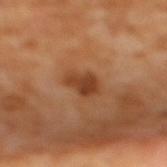image: ~15 mm tile from a whole-body skin photo; location: the upper back; illumination: cross-polarized; subject: female, about 55 years old; lesion size: ~3 mm (longest diameter).